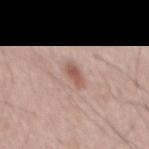Part of a total-body skin-imaging series; this lesion was reviewed on a skin check and was not flagged for biopsy.
The patient is a male in their mid-50s.
The tile uses white-light illumination.
A close-up tile cropped from a whole-body skin photograph, about 15 mm across.
Approximately 3 mm at its widest.